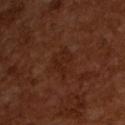• biopsy status · total-body-photography surveillance lesion; no biopsy
• image-analysis metrics · a footprint of about 5.5 mm² and an outline eccentricity of about 0.65 (0 = round, 1 = elongated); a border-irregularity index near 5/10, a within-lesion color-variation index near 1.5/10, and peripheral color asymmetry of about 0.5; a nevus-likeness score of about 10/100 and a lesion-detection confidence of about 100/100
• illumination · cross-polarized
• image source · ~15 mm crop, total-body skin-cancer survey
• subject · male, aged 63–67
• size · ≈3 mm